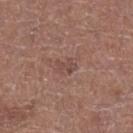patient = male, approximately 75 years of age
body site = the leg
acquisition = 15 mm crop, total-body photography